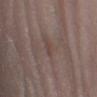Impression:
The lesion was tiled from a total-body skin photograph and was not biopsied.
Context:
Cropped from a total-body skin-imaging series; the visible field is about 15 mm. From the left lower leg. A male subject, in their mid- to late 60s. The total-body-photography lesion software estimated an area of roughly 2 mm², a shape eccentricity near 0.75, and a symmetry-axis asymmetry near 0.35. It also reported an average lesion color of about L≈43 a*≈15 b*≈20 (CIELAB), about 5 CIELAB-L* units darker than the surrounding skin, and a normalized border contrast of about 4.5. The analysis additionally found a border-irregularity rating of about 2.5/10 and peripheral color asymmetry of about 0. And it measured a nevus-likeness score of about 0/100. The recorded lesion diameter is about 1.5 mm. Imaged with white-light lighting.